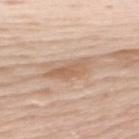– image source · total-body-photography crop, ~15 mm field of view
– body site · the upper back
– patient · female, aged approximately 50